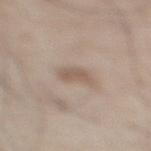This lesion was catalogued during total-body skin photography and was not selected for biopsy.
The lesion is on the right lower leg.
A lesion tile, about 15 mm wide, cut from a 3D total-body photograph.
A male subject aged 58–62.